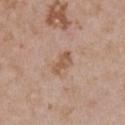Imaged during a routine full-body skin examination; the lesion was not biopsied and no histopathology is available. A female patient, aged around 30. Cropped from a whole-body photographic skin survey; the tile spans about 15 mm. About 3 mm across. This is a white-light tile. Located on the front of the torso. An algorithmic analysis of the crop reported a lesion color around L≈55 a*≈19 b*≈31 in CIELAB, roughly 9 lightness units darker than nearby skin, and a lesion-to-skin contrast of about 7 (normalized; higher = more distinct). The analysis additionally found a border-irregularity rating of about 4/10 and a color-variation rating of about 2/10. The software also gave an automated nevus-likeness rating near 5 out of 100 and a lesion-detection confidence of about 100/100.